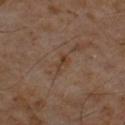Context:
Automated image analysis of the tile measured roughly 6 lightness units darker than nearby skin and a lesion-to-skin contrast of about 6.5 (normalized; higher = more distinct). A region of skin cropped from a whole-body photographic capture, roughly 15 mm wide. The tile uses cross-polarized illumination. Longest diameter approximately 2.5 mm. The lesion is on the front of the torso. A male subject aged 58 to 62.